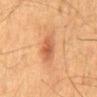No biopsy was performed on this lesion — it was imaged during a full skin examination and was not determined to be concerning.
The total-body-photography lesion software estimated a lesion color around L≈48 a*≈23 b*≈33 in CIELAB, a lesion–skin lightness drop of about 9, and a lesion-to-skin contrast of about 7 (normalized; higher = more distinct). The software also gave a border-irregularity index near 2.5/10, a within-lesion color-variation index near 3.5/10, and peripheral color asymmetry of about 1.
This image is a 15 mm lesion crop taken from a total-body photograph.
The subject is a male about 65 years old.
On the mid back.
The tile uses cross-polarized illumination.
Longest diameter approximately 3 mm.